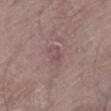<record>
<biopsy_status>not biopsied; imaged during a skin examination</biopsy_status>
<site>right thigh</site>
<image>
  <source>total-body photography crop</source>
  <field_of_view_mm>15</field_of_view_mm>
</image>
<patient>
  <sex>male</sex>
  <age_approx>65</age_approx>
</patient>
<lighting>white-light</lighting>
</record>